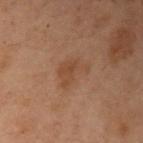Impression: The lesion was photographed on a routine skin check and not biopsied; there is no pathology result. Context: A female subject aged 53 to 57. The tile uses cross-polarized illumination. Measured at roughly 4 mm in maximum diameter. A region of skin cropped from a whole-body photographic capture, roughly 15 mm wide. The lesion is on the left arm.